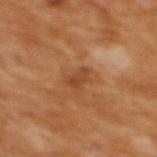The lesion was tiled from a total-body skin photograph and was not biopsied.
A close-up tile cropped from a whole-body skin photograph, about 15 mm across.
A female patient aged approximately 55.
On the upper back.